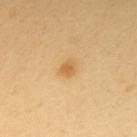Assessment:
Recorded during total-body skin imaging; not selected for excision or biopsy.
Acquisition and patient details:
From the back. A 15 mm crop from a total-body photograph taken for skin-cancer surveillance. A female patient aged 53 to 57. The total-body-photography lesion software estimated a lesion area of about 3 mm², a shape eccentricity near 0.55, and two-axis asymmetry of about 0.3. It also reported a mean CIELAB color near L≈63 a*≈20 b*≈46, a lesion–skin lightness drop of about 9, and a normalized border contrast of about 7. It also reported a border-irregularity index near 2.5/10 and internal color variation of about 1.5 on a 0–10 scale.